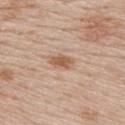No biopsy was performed on this lesion — it was imaged during a full skin examination and was not determined to be concerning.
The lesion is on the upper back.
Automated image analysis of the tile measured a classifier nevus-likeness of about 90/100.
A 15 mm crop from a total-body photograph taken for skin-cancer surveillance.
A female subject approximately 50 years of age.